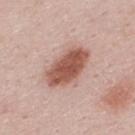Recorded during total-body skin imaging; not selected for excision or biopsy. The lesion is on the upper back. A 15 mm close-up extracted from a 3D total-body photography capture. The patient is a male about 25 years old. The tile uses white-light illumination. About 6 mm across. The lesion-visualizer software estimated an eccentricity of roughly 0.8 and two-axis asymmetry of about 0.15. And it measured a mean CIELAB color near L≈54 a*≈23 b*≈27, a lesion–skin lightness drop of about 15, and a lesion-to-skin contrast of about 10.5 (normalized; higher = more distinct). It also reported border irregularity of about 2 on a 0–10 scale and a color-variation rating of about 3.5/10. The software also gave an automated nevus-likeness rating near 100 out of 100 and a lesion-detection confidence of about 100/100.